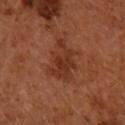| field | value |
|---|---|
| notes | total-body-photography surveillance lesion; no biopsy |
| anatomic site | the arm |
| image | ~15 mm crop, total-body skin-cancer survey |
| patient | female, approximately 65 years of age |